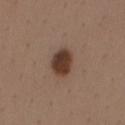Assessment: No biopsy was performed on this lesion — it was imaged during a full skin examination and was not determined to be concerning. Acquisition and patient details: A lesion tile, about 15 mm wide, cut from a 3D total-body photograph. About 3.5 mm across. The lesion is on the mid back. A male patient in their 40s.